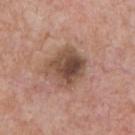| key | value |
|---|---|
| image | ~15 mm tile from a whole-body skin photo |
| diameter | ≈4 mm |
| tile lighting | white-light |
| automated metrics | an eccentricity of roughly 0.15; lesion-presence confidence of about 100/100 |
| site | the chest |
| patient | male, aged approximately 75 |